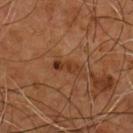Clinical summary: Imaged with cross-polarized lighting. A 15 mm crop from a total-body photograph taken for skin-cancer surveillance. Located on the chest. The patient is a male aged around 55.